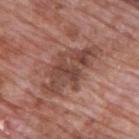workup=total-body-photography surveillance lesion; no biopsy | image-analysis metrics=a classifier nevus-likeness of about 10/100 | imaging modality=15 mm crop, total-body photography | location=the upper back | subject=male, approximately 70 years of age | lighting=white-light.